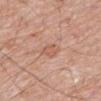Impression:
Imaged during a routine full-body skin examination; the lesion was not biopsied and no histopathology is available.
Image and clinical context:
This is a white-light tile. Measured at roughly 3 mm in maximum diameter. The patient is a male aged around 80. On the mid back. Cropped from a whole-body photographic skin survey; the tile spans about 15 mm.